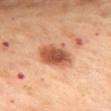This is a cross-polarized tile. The patient is a female aged 53–57. From the mid back. A close-up tile cropped from a whole-body skin photograph, about 15 mm across.